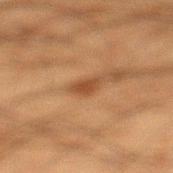acquisition = ~15 mm crop, total-body skin-cancer survey; subject = male, roughly 35 years of age; location = the left lower leg.